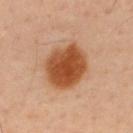Imaged during a routine full-body skin examination; the lesion was not biopsied and no histopathology is available.
Measured at roughly 5.5 mm in maximum diameter.
The tile uses cross-polarized illumination.
On the upper back.
A male subject roughly 45 years of age.
An algorithmic analysis of the crop reported an eccentricity of roughly 0.3 and two-axis asymmetry of about 0.15. And it measured roughly 15 lightness units darker than nearby skin and a lesion-to-skin contrast of about 11.5 (normalized; higher = more distinct). The software also gave a border-irregularity rating of about 1.5/10, a color-variation rating of about 4.5/10, and radial color variation of about 1.5. And it measured an automated nevus-likeness rating near 100 out of 100 and a detector confidence of about 100 out of 100 that the crop contains a lesion.
A 15 mm crop from a total-body photograph taken for skin-cancer surveillance.